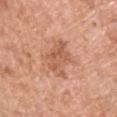Clinical impression: The lesion was photographed on a routine skin check and not biopsied; there is no pathology result. Clinical summary: The subject is a male aged 53 to 57. Approximately 4.5 mm at its widest. The lesion is located on the chest. This is a white-light tile. A 15 mm close-up tile from a total-body photography series done for melanoma screening.